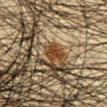{"biopsy_status": "not biopsied; imaged during a skin examination", "site": "front of the torso", "patient": {"sex": "male", "age_approx": 45}, "image": {"source": "total-body photography crop", "field_of_view_mm": 15}, "lighting": "cross-polarized", "lesion_size": {"long_diameter_mm_approx": 2.5}}